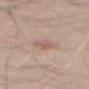The subject is a male in their 50s. About 3.5 mm across. From the back. Automated image analysis of the tile measured a lesion color around L≈59 a*≈18 b*≈25 in CIELAB, roughly 8 lightness units darker than nearby skin, and a normalized border contrast of about 5. The analysis additionally found a border-irregularity rating of about 2.5/10, internal color variation of about 2.5 on a 0–10 scale, and a peripheral color-asymmetry measure near 1. The software also gave a nevus-likeness score of about 0/100 and lesion-presence confidence of about 100/100. A region of skin cropped from a whole-body photographic capture, roughly 15 mm wide.